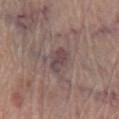Imaged during a routine full-body skin examination; the lesion was not biopsied and no histopathology is available. The lesion is on the left lower leg. This is a white-light tile. A roughly 15 mm field-of-view crop from a total-body skin photograph. A male patient approximately 70 years of age.